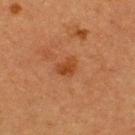Captured during whole-body skin photography for melanoma surveillance; the lesion was not biopsied. The lesion's longest dimension is about 3 mm. The lesion-visualizer software estimated a lesion area of about 4.5 mm², an eccentricity of roughly 0.65, and a shape-asymmetry score of about 0.35 (0 = symmetric). The software also gave a lesion color around L≈37 a*≈23 b*≈34 in CIELAB and a lesion–skin lightness drop of about 7. The analysis additionally found a border-irregularity index near 3/10, a color-variation rating of about 2.5/10, and radial color variation of about 1. It also reported a nevus-likeness score of about 25/100 and a detector confidence of about 100 out of 100 that the crop contains a lesion. The lesion is located on the upper back. A 15 mm close-up extracted from a 3D total-body photography capture. The subject is a male aged 38–42.